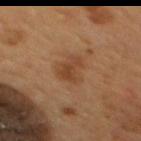Clinical impression:
Captured during whole-body skin photography for melanoma surveillance; the lesion was not biopsied.
Acquisition and patient details:
Located on the mid back. A male subject approximately 55 years of age. Cropped from a whole-body photographic skin survey; the tile spans about 15 mm.